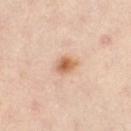| feature | finding |
|---|---|
| biopsy status | total-body-photography surveillance lesion; no biopsy |
| illumination | cross-polarized |
| body site | the right leg |
| subject | female, in their 40s |
| lesion diameter | about 2.5 mm |
| acquisition | ~15 mm tile from a whole-body skin photo |